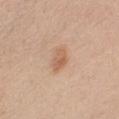Clinical impression: No biopsy was performed on this lesion — it was imaged during a full skin examination and was not determined to be concerning. Background: The lesion's longest dimension is about 3 mm. A close-up tile cropped from a whole-body skin photograph, about 15 mm across. Located on the chest. Captured under white-light illumination. The subject is a female about 40 years old.